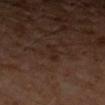The recorded lesion diameter is about 2.5 mm.
A 15 mm close-up extracted from a 3D total-body photography capture.
Captured under cross-polarized illumination.
On the arm.
The patient is a male aged approximately 60.